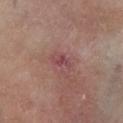<case>
  <biopsy_status>not biopsied; imaged during a skin examination</biopsy_status>
  <image>
    <source>total-body photography crop</source>
    <field_of_view_mm>15</field_of_view_mm>
  </image>
  <lesion_size>
    <long_diameter_mm_approx>2.5</long_diameter_mm_approx>
  </lesion_size>
  <patient>
    <sex>male</sex>
    <age_approx>65</age_approx>
  </patient>
  <automated_metrics>
    <area_mm2_approx>3.0</area_mm2_approx>
    <eccentricity>0.85</eccentricity>
    <shape_asymmetry>0.5</shape_asymmetry>
    <cielab_L>42</cielab_L>
    <cielab_a>25</cielab_a>
    <cielab_b>18</cielab_b>
    <vs_skin_darker_L>7.0</vs_skin_darker_L>
    <vs_skin_contrast_norm>6.5</vs_skin_contrast_norm>
    <border_irregularity_0_10>5.0</border_irregularity_0_10>
    <color_variation_0_10>0.5</color_variation_0_10>
    <peripheral_color_asymmetry>0.0</peripheral_color_asymmetry>
    <nevus_likeness_0_100>0</nevus_likeness_0_100>
  </automated_metrics>
  <lighting>cross-polarized</lighting>
  <site>left lower leg</site>
</case>